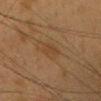Assessment:
Imaged during a routine full-body skin examination; the lesion was not biopsied and no histopathology is available.
Image and clinical context:
The recorded lesion diameter is about 3 mm. The lesion is located on the head or neck. The tile uses cross-polarized illumination. Automated tile analysis of the lesion measured a lesion area of about 4.5 mm² and two-axis asymmetry of about 0.2. The software also gave an automated nevus-likeness rating near 5 out of 100 and a lesion-detection confidence of about 100/100. Cropped from a total-body skin-imaging series; the visible field is about 15 mm. A female subject, in their 40s.